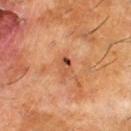Impression: Captured during whole-body skin photography for melanoma surveillance; the lesion was not biopsied. Clinical summary: The lesion is on the right lower leg. The tile uses cross-polarized illumination. A male subject about 60 years old. Automated tile analysis of the lesion measured a shape-asymmetry score of about 0.25 (0 = symmetric). It also reported a detector confidence of about 100 out of 100 that the crop contains a lesion. Cropped from a total-body skin-imaging series; the visible field is about 15 mm.